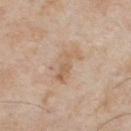Impression: Recorded during total-body skin imaging; not selected for excision or biopsy. Image and clinical context: The patient is a male aged 53 to 57. This image is a 15 mm lesion crop taken from a total-body photograph. Imaged with white-light lighting. From the chest. The recorded lesion diameter is about 4.5 mm.